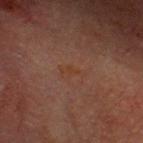This lesion was catalogued during total-body skin photography and was not selected for biopsy. The patient is a male about 75 years old. Approximately 2.5 mm at its widest. Located on the head or neck. A 15 mm crop from a total-body photograph taken for skin-cancer surveillance. The tile uses cross-polarized illumination.